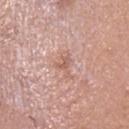Cropped from a total-body skin-imaging series; the visible field is about 15 mm. A female patient aged 63–67. Measured at roughly 3 mm in maximum diameter. The lesion-visualizer software estimated an average lesion color of about L≈60 a*≈22 b*≈27 (CIELAB) and a lesion-to-skin contrast of about 5.5 (normalized; higher = more distinct). From the head or neck. Imaged with white-light lighting.